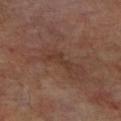Clinical impression: Captured during whole-body skin photography for melanoma surveillance; the lesion was not biopsied. Background: The lesion-visualizer software estimated internal color variation of about 0 on a 0–10 scale and radial color variation of about 0. A male patient, aged 68–72. The lesion is on the leg. A roughly 15 mm field-of-view crop from a total-body skin photograph. This is a cross-polarized tile. The recorded lesion diameter is about 3.5 mm.